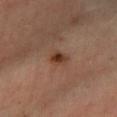biopsy status: total-body-photography surveillance lesion; no biopsy | diameter: about 2.5 mm | location: the left lower leg | acquisition: 15 mm crop, total-body photography | image-analysis metrics: a mean CIELAB color near L≈39 a*≈21 b*≈29, a lesion–skin lightness drop of about 10, and a normalized lesion–skin contrast near 8.5; a border-irregularity rating of about 2/10, internal color variation of about 4.5 on a 0–10 scale, and peripheral color asymmetry of about 1.5; an automated nevus-likeness rating near 90 out of 100 and lesion-presence confidence of about 100/100 | illumination: cross-polarized | subject: female, aged 58 to 62.